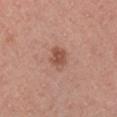| key | value |
|---|---|
| size | ≈3 mm |
| subject | male, aged approximately 40 |
| body site | the arm |
| lighting | white-light |
| image source | 15 mm crop, total-body photography |